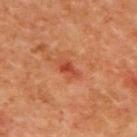  biopsy_status: not biopsied; imaged during a skin examination
  lesion_size:
    long_diameter_mm_approx: 3.0
  lighting: cross-polarized
  site: back
  image:
    source: total-body photography crop
    field_of_view_mm: 15
  patient:
    sex: female
    age_approx: 40
  automated_metrics:
    cielab_L: 49
    cielab_a: 34
    cielab_b: 38
    vs_skin_contrast_norm: 6.5
    lesion_detection_confidence_0_100: 100